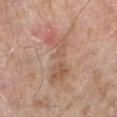Impression: Captured during whole-body skin photography for melanoma surveillance; the lesion was not biopsied. Acquisition and patient details: A male patient, in their 80s. Captured under white-light illumination. A roughly 15 mm field-of-view crop from a total-body skin photograph. The lesion-visualizer software estimated a lesion area of about 13 mm², an eccentricity of roughly 0.9, and two-axis asymmetry of about 0.5. It also reported a border-irregularity index near 9/10, a color-variation rating of about 4.5/10, and radial color variation of about 1. The analysis additionally found lesion-presence confidence of about 100/100. The lesion is located on the left lower leg.